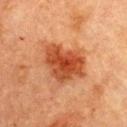Captured during whole-body skin photography for melanoma surveillance; the lesion was not biopsied. Longest diameter approximately 6 mm. Captured under cross-polarized illumination. On the chest. A roughly 15 mm field-of-view crop from a total-body skin photograph. A female patient aged around 60.